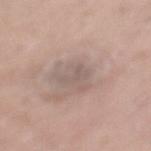Q: Was a biopsy performed?
A: no biopsy performed (imaged during a skin exam)
Q: What are the patient's age and sex?
A: female, in their mid- to late 60s
Q: Automated lesion metrics?
A: border irregularity of about 6.5 on a 0–10 scale; a classifier nevus-likeness of about 0/100
Q: What is the imaging modality?
A: ~15 mm tile from a whole-body skin photo
Q: How large is the lesion?
A: about 3 mm
Q: Lesion location?
A: the mid back
Q: How was the tile lit?
A: white-light